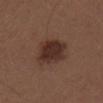  biopsy_status: not biopsied; imaged during a skin examination
  lesion_size:
    long_diameter_mm_approx: 4.0
  lighting: white-light
  site: arm
  patient:
    sex: male
    age_approx: 30
  image:
    source: total-body photography crop
    field_of_view_mm: 15